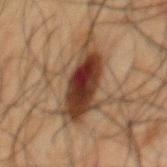Recorded during total-body skin imaging; not selected for excision or biopsy.
About 9 mm across.
A roughly 15 mm field-of-view crop from a total-body skin photograph.
A male patient roughly 50 years of age.
Captured under cross-polarized illumination.
Located on the back.